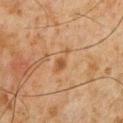Recorded during total-body skin imaging; not selected for excision or biopsy. This image is a 15 mm lesion crop taken from a total-body photograph. The lesion-visualizer software estimated a footprint of about 4.5 mm², an outline eccentricity of about 0.9 (0 = round, 1 = elongated), and a symmetry-axis asymmetry near 0.35. The software also gave an average lesion color of about L≈46 a*≈18 b*≈33 (CIELAB), about 6 CIELAB-L* units darker than the surrounding skin, and a normalized border contrast of about 5.5. The analysis additionally found a border-irregularity rating of about 4/10 and peripheral color asymmetry of about 0.5. The software also gave lesion-presence confidence of about 100/100. Captured under cross-polarized illumination. A male patient aged approximately 60. The lesion's longest dimension is about 4 mm. The lesion is on the chest.